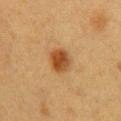Image and clinical context:
A 15 mm close-up tile from a total-body photography series done for melanoma screening. The lesion is on the chest. The lesion's longest dimension is about 3 mm. An algorithmic analysis of the crop reported a mean CIELAB color near L≈39 a*≈20 b*≈34, roughly 11 lightness units darker than nearby skin, and a normalized lesion–skin contrast near 9.5. And it measured a border-irregularity index near 1.5/10, a within-lesion color-variation index near 3.5/10, and radial color variation of about 1. A female patient approximately 40 years of age. Imaged with cross-polarized lighting.